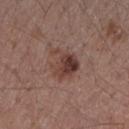Q: Was this lesion biopsied?
A: imaged on a skin check; not biopsied
Q: What kind of image is this?
A: ~15 mm crop, total-body skin-cancer survey
Q: Illumination type?
A: white-light
Q: What did automated image analysis measure?
A: a lesion area of about 9.5 mm², an outline eccentricity of about 0.55 (0 = round, 1 = elongated), and a shape-asymmetry score of about 0.4 (0 = symmetric); a lesion color around L≈40 a*≈19 b*≈23 in CIELAB, roughly 10 lightness units darker than nearby skin, and a normalized lesion–skin contrast near 8.5; an automated nevus-likeness rating near 85 out of 100
Q: Patient demographics?
A: female, aged 53–57
Q: Lesion location?
A: the right forearm